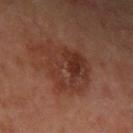{
  "patient": {
    "sex": "male",
    "age_approx": 65
  },
  "site": "left upper arm",
  "image": {
    "source": "total-body photography crop",
    "field_of_view_mm": 15
  }
}